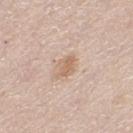The lesion was tiled from a total-body skin photograph and was not biopsied. A roughly 15 mm field-of-view crop from a total-body skin photograph. From the left thigh. A female patient about 45 years old. The lesion's longest dimension is about 3 mm.